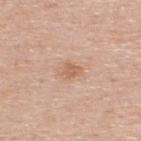Q: Illumination type?
A: white-light
Q: Where on the body is the lesion?
A: the upper back
Q: How was this image acquired?
A: ~15 mm tile from a whole-body skin photo
Q: Who is the patient?
A: male, roughly 65 years of age